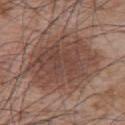<lesion>
<biopsy_status>not biopsied; imaged during a skin examination</biopsy_status>
<patient>
  <sex>male</sex>
  <age_approx>65</age_approx>
</patient>
<lesion_size>
  <long_diameter_mm_approx>10.0</long_diameter_mm_approx>
</lesion_size>
<automated_metrics>
  <area_mm2_approx>45.0</area_mm2_approx>
  <eccentricity>0.75</eccentricity>
  <shape_asymmetry>0.2</shape_asymmetry>
  <cielab_L>45</cielab_L>
  <cielab_a>19</cielab_a>
  <cielab_b>24</cielab_b>
  <vs_skin_contrast_norm>7.5</vs_skin_contrast_norm>
</automated_metrics>
<site>upper back</site>
<lighting>white-light</lighting>
<image>
  <source>total-body photography crop</source>
  <field_of_view_mm>15</field_of_view_mm>
</image>
</lesion>